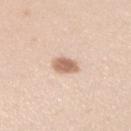biopsy status=imaged on a skin check; not biopsied
size=~3 mm (longest diameter)
automated lesion analysis=an average lesion color of about L≈65 a*≈19 b*≈30 (CIELAB) and roughly 14 lightness units darker than nearby skin
image source=15 mm crop, total-body photography
illumination=white-light
anatomic site=the arm
patient=female, approximately 20 years of age